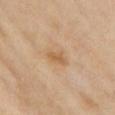On the chest.
This image is a 15 mm lesion crop taken from a total-body photograph.
A female subject roughly 40 years of age.